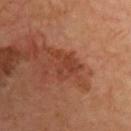notes — catalogued during a skin exam; not biopsied
image source — ~15 mm crop, total-body skin-cancer survey
lighting — cross-polarized
location — the front of the torso
lesion size — ~3.5 mm (longest diameter)
patient — male, aged 63 to 67